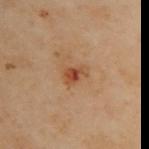No biopsy was performed on this lesion — it was imaged during a full skin examination and was not determined to be concerning. A 15 mm crop from a total-body photograph taken for skin-cancer surveillance. The patient is a female in their 60s. The lesion is located on the upper back.